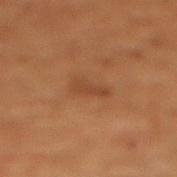Part of a total-body skin-imaging series; this lesion was reviewed on a skin check and was not flagged for biopsy.
Approximately 3 mm at its widest.
An algorithmic analysis of the crop reported a lesion area of about 4.5 mm² and a shape eccentricity near 0.85. The analysis additionally found a mean CIELAB color near L≈32 a*≈18 b*≈27, a lesion–skin lightness drop of about 5, and a normalized lesion–skin contrast near 5. The analysis additionally found border irregularity of about 4 on a 0–10 scale, a color-variation rating of about 1/10, and peripheral color asymmetry of about 0.5.
A 15 mm close-up extracted from a 3D total-body photography capture.
The subject is a female about 80 years old.
On the chest.
This is a cross-polarized tile.